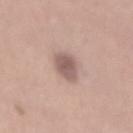Q: How was this image acquired?
A: ~15 mm tile from a whole-body skin photo
Q: What did automated image analysis measure?
A: a lesion area of about 7.5 mm² and an outline eccentricity of about 0.8 (0 = round, 1 = elongated); a classifier nevus-likeness of about 15/100
Q: Who is the patient?
A: male, approximately 45 years of age
Q: Lesion location?
A: the mid back
Q: What is the lesion's diameter?
A: about 4 mm